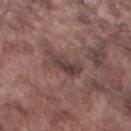{"biopsy_status": "not biopsied; imaged during a skin examination", "site": "left thigh", "lighting": "white-light", "image": {"source": "total-body photography crop", "field_of_view_mm": 15}, "patient": {"sex": "male", "age_approx": 75}, "lesion_size": {"long_diameter_mm_approx": 4.0}}